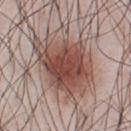notes: imaged on a skin check; not biopsied | location: the chest | image-analysis metrics: an area of roughly 33 mm², an outline eccentricity of about 0.7 (0 = round, 1 = elongated), and two-axis asymmetry of about 0.3; an average lesion color of about L≈48 a*≈20 b*≈23 (CIELAB), roughly 14 lightness units darker than nearby skin, and a normalized lesion–skin contrast near 10 | tile lighting: white-light illumination | subject: male, aged approximately 35 | acquisition: ~15 mm tile from a whole-body skin photo.